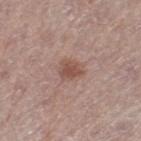workup: total-body-photography surveillance lesion; no biopsy | imaging modality: total-body-photography crop, ~15 mm field of view | body site: the right thigh | patient: female, aged 63 to 67 | illumination: white-light illumination.